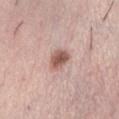Impression: The lesion was tiled from a total-body skin photograph and was not biopsied. Image and clinical context: A female subject, in their mid- to late 60s. A roughly 15 mm field-of-view crop from a total-body skin photograph. On the abdomen. The recorded lesion diameter is about 3 mm. Automated image analysis of the tile measured an eccentricity of roughly 0.7 and two-axis asymmetry of about 0.25. It also reported an average lesion color of about L≈56 a*≈21 b*≈25 (CIELAB) and a lesion-to-skin contrast of about 9.5 (normalized; higher = more distinct).